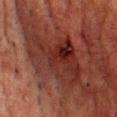Imaged during a routine full-body skin examination; the lesion was not biopsied and no histopathology is available. Captured under cross-polarized illumination. Longest diameter approximately 9 mm. A region of skin cropped from a whole-body photographic capture, roughly 15 mm wide. The lesion is located on the chest. The patient is a male aged 58 to 62.